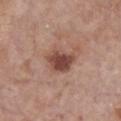Case summary:
* notes: total-body-photography surveillance lesion; no biopsy
* imaging modality: ~15 mm tile from a whole-body skin photo
* patient: female, approximately 85 years of age or older
* anatomic site: the chest